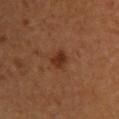A female subject aged 33–37.
Located on the upper back.
A lesion tile, about 15 mm wide, cut from a 3D total-body photograph.
This is a cross-polarized tile.